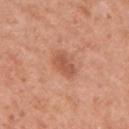Notes:
– workup: no biopsy performed (imaged during a skin exam)
– image source: total-body-photography crop, ~15 mm field of view
– site: the right upper arm
– subject: female, approximately 50 years of age